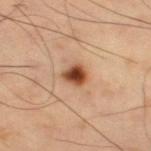notes — total-body-photography surveillance lesion; no biopsy | tile lighting — cross-polarized | image source — ~15 mm crop, total-body skin-cancer survey | patient — male, aged 58 to 62 | anatomic site — the right thigh | automated lesion analysis — a shape eccentricity near 0.4 and a shape-asymmetry score of about 0.25 (0 = symmetric); a mean CIELAB color near L≈48 a*≈24 b*≈34, about 17 CIELAB-L* units darker than the surrounding skin, and a lesion-to-skin contrast of about 12 (normalized; higher = more distinct); border irregularity of about 2 on a 0–10 scale, internal color variation of about 6.5 on a 0–10 scale, and radial color variation of about 1.5.